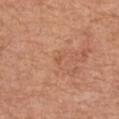Part of a total-body skin-imaging series; this lesion was reviewed on a skin check and was not flagged for biopsy.
The lesion is on the left forearm.
A roughly 15 mm field-of-view crop from a total-body skin photograph.
An algorithmic analysis of the crop reported a lesion area of about 1 mm², an eccentricity of roughly 0.6, and a shape-asymmetry score of about 0.45 (0 = symmetric). The software also gave peripheral color asymmetry of about 0.
Captured under white-light illumination.
Measured at roughly 1 mm in maximum diameter.
A female patient aged 48–52.